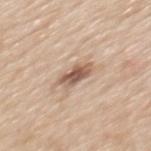The lesion was photographed on a routine skin check and not biopsied; there is no pathology result. Longest diameter approximately 3.5 mm. A close-up tile cropped from a whole-body skin photograph, about 15 mm across. Located on the mid back. The subject is a male aged 58 to 62.